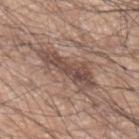The tile uses white-light illumination. Automated image analysis of the tile measured a shape-asymmetry score of about 0.4 (0 = symmetric). And it measured an average lesion color of about L≈49 a*≈17 b*≈24 (CIELAB), a lesion–skin lightness drop of about 11, and a lesion-to-skin contrast of about 8 (normalized; higher = more distinct). The software also gave an automated nevus-likeness rating near 30 out of 100 and a lesion-detection confidence of about 65/100. Cropped from a total-body skin-imaging series; the visible field is about 15 mm. A male subject approximately 70 years of age. Longest diameter approximately 7 mm. On the left upper arm.